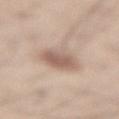The lesion was tiled from a total-body skin photograph and was not biopsied. Captured under white-light illumination. Measured at roughly 4 mm in maximum diameter. A male subject aged around 55. A lesion tile, about 15 mm wide, cut from a 3D total-body photograph. On the lower back.